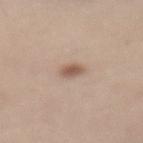biopsy status = no biopsy performed (imaged during a skin exam) | image source = ~15 mm crop, total-body skin-cancer survey | body site = the back | subject = female, roughly 65 years of age | size = about 2.5 mm.